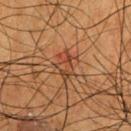This lesion was catalogued during total-body skin photography and was not selected for biopsy.
From the chest.
Captured under cross-polarized illumination.
Cropped from a total-body skin-imaging series; the visible field is about 15 mm.
The subject is a male about 55 years old.
The lesion's longest dimension is about 3.5 mm.